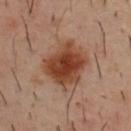Case summary:
• workup · catalogued during a skin exam; not biopsied
• location · the upper back
• image · ~15 mm crop, total-body skin-cancer survey
• subject · male, about 40 years old